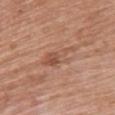Impression: Recorded during total-body skin imaging; not selected for excision or biopsy. Image and clinical context: Imaged with white-light lighting. A close-up tile cropped from a whole-body skin photograph, about 15 mm across. The lesion is located on the upper back. A female subject aged around 65. The recorded lesion diameter is about 5.5 mm.